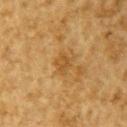notes — no biopsy performed (imaged during a skin exam)
imaging modality — total-body-photography crop, ~15 mm field of view
illumination — cross-polarized
body site — the right upper arm
patient — male, in their mid- to late 80s or older